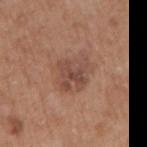- notes · total-body-photography surveillance lesion; no biopsy
- diameter · ≈4.5 mm
- anatomic site · the right upper arm
- image · ~15 mm crop, total-body skin-cancer survey
- tile lighting · white-light illumination
- TBP lesion metrics · an outline eccentricity of about 0.6 (0 = round, 1 = elongated) and a symmetry-axis asymmetry near 0.25; a mean CIELAB color near L≈48 a*≈21 b*≈26, a lesion–skin lightness drop of about 8, and a normalized lesion–skin contrast near 6.5; a border-irregularity index near 3/10 and internal color variation of about 5 on a 0–10 scale; a nevus-likeness score of about 15/100 and a lesion-detection confidence of about 100/100
- patient · male, aged around 65